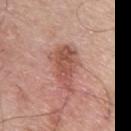workup=no biopsy performed (imaged during a skin exam)
lighting=white-light illumination
subject=male, in their mid-60s
acquisition=total-body-photography crop, ~15 mm field of view
automated metrics=a classifier nevus-likeness of about 65/100 and a detector confidence of about 100 out of 100 that the crop contains a lesion
site=the left upper arm
size=≈5.5 mm